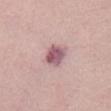Q: Was a biopsy performed?
A: no biopsy performed (imaged during a skin exam)
Q: What is the anatomic site?
A: the chest
Q: What is the lesion's diameter?
A: ~3 mm (longest diameter)
Q: What lighting was used for the tile?
A: white-light illumination
Q: What is the imaging modality?
A: total-body-photography crop, ~15 mm field of view
Q: Patient demographics?
A: female, approximately 50 years of age